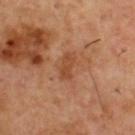Captured during whole-body skin photography for melanoma surveillance; the lesion was not biopsied.
On the back.
About 3 mm across.
Captured under cross-polarized illumination.
An algorithmic analysis of the crop reported border irregularity of about 2.5 on a 0–10 scale and peripheral color asymmetry of about 0.5. The software also gave a classifier nevus-likeness of about 0/100 and a detector confidence of about 100 out of 100 that the crop contains a lesion.
A lesion tile, about 15 mm wide, cut from a 3D total-body photograph.
The subject is a male in their mid-50s.